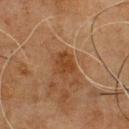Case summary:
– notes · imaged on a skin check; not biopsied
– diameter · about 3 mm
– TBP lesion metrics · a mean CIELAB color near L≈33 a*≈19 b*≈30 and a normalized lesion–skin contrast near 7.5; a border-irregularity rating of about 1.5/10, a within-lesion color-variation index near 2/10, and peripheral color asymmetry of about 0.5
– image · ~15 mm tile from a whole-body skin photo
– site · the chest
– patient · male, approximately 75 years of age